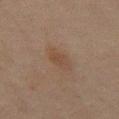biopsy status: no biopsy performed (imaged during a skin exam)
illumination: cross-polarized illumination
acquisition: ~15 mm tile from a whole-body skin photo
subject: male, approximately 45 years of age
TBP lesion metrics: a lesion color around L≈36 a*≈14 b*≈23 in CIELAB, about 5 CIELAB-L* units darker than the surrounding skin, and a lesion-to-skin contrast of about 5 (normalized; higher = more distinct); a border-irregularity index near 2.5/10 and internal color variation of about 2 on a 0–10 scale; a nevus-likeness score of about 15/100 and lesion-presence confidence of about 100/100
body site: the mid back